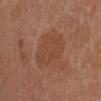Clinical impression: Imaged during a routine full-body skin examination; the lesion was not biopsied and no histopathology is available. Context: Captured under white-light illumination. Measured at roughly 5.5 mm in maximum diameter. A 15 mm close-up tile from a total-body photography series done for melanoma screening. An algorithmic analysis of the crop reported a lesion area of about 15 mm², a shape eccentricity near 0.75, and two-axis asymmetry of about 0.2. The software also gave a border-irregularity index near 2/10 and radial color variation of about 0.5. It also reported an automated nevus-likeness rating near 5 out of 100 and a lesion-detection confidence of about 100/100. A female patient roughly 65 years of age. Located on the left leg.